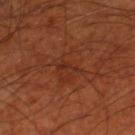Impression:
Imaged during a routine full-body skin examination; the lesion was not biopsied and no histopathology is available.
Image and clinical context:
The subject is a male about 70 years old. A 15 mm crop from a total-body photograph taken for skin-cancer surveillance. Measured at roughly 2.5 mm in maximum diameter. Located on the right thigh. The tile uses cross-polarized illumination.